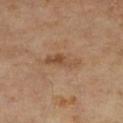{"biopsy_status": "not biopsied; imaged during a skin examination", "image": {"source": "total-body photography crop", "field_of_view_mm": 15}, "lesion_size": {"long_diameter_mm_approx": 4.5}, "site": "left lower leg", "patient": {"sex": "female", "age_approx": 75}, "automated_metrics": {"color_variation_0_10": 4.5, "peripheral_color_asymmetry": 1.5, "nevus_likeness_0_100": 0, "lesion_detection_confidence_0_100": 100}}